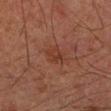<lesion>
  <biopsy_status>not biopsied; imaged during a skin examination</biopsy_status>
  <site>right forearm</site>
  <patient>
    <sex>male</sex>
    <age_approx>65</age_approx>
  </patient>
  <image>
    <source>total-body photography crop</source>
    <field_of_view_mm>15</field_of_view_mm>
  </image>
</lesion>